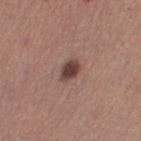{"biopsy_status": "not biopsied; imaged during a skin examination", "site": "left thigh", "image": {"source": "total-body photography crop", "field_of_view_mm": 15}, "patient": {"sex": "female", "age_approx": 30}, "lesion_size": {"long_diameter_mm_approx": 3.0}, "lighting": "white-light"}